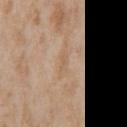Recorded during total-body skin imaging; not selected for excision or biopsy.
A male subject, aged around 65.
Located on the front of the torso.
This is a white-light tile.
Longest diameter approximately 3 mm.
A region of skin cropped from a whole-body photographic capture, roughly 15 mm wide.